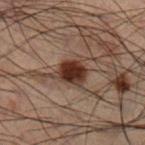  biopsy_status: not biopsied; imaged during a skin examination
  patient:
    sex: male
    age_approx: 50
  image:
    source: total-body photography crop
    field_of_view_mm: 15
  site: leg